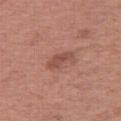follow-up — total-body-photography surveillance lesion; no biopsy
site — the right thigh
imaging modality — ~15 mm crop, total-body skin-cancer survey
subject — female, roughly 40 years of age